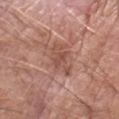| feature | finding |
|---|---|
| illumination | white-light |
| subject | male, about 65 years old |
| site | the left forearm |
| imaging modality | total-body-photography crop, ~15 mm field of view |
| size | ≈3 mm |